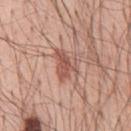Q: Is there a histopathology result?
A: total-body-photography surveillance lesion; no biopsy
Q: Patient demographics?
A: male, aged 53–57
Q: Illumination type?
A: white-light
Q: What is the anatomic site?
A: the front of the torso
Q: What is the lesion's diameter?
A: ≈4 mm
Q: What did automated image analysis measure?
A: a footprint of about 7.5 mm², an outline eccentricity of about 0.75 (0 = round, 1 = elongated), and two-axis asymmetry of about 0.5; a peripheral color-asymmetry measure near 1.5; a lesion-detection confidence of about 100/100
Q: What kind of image is this?
A: total-body-photography crop, ~15 mm field of view